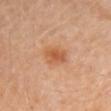This lesion was catalogued during total-body skin photography and was not selected for biopsy. On the abdomen. A male subject, about 60 years old. An algorithmic analysis of the crop reported an area of roughly 6 mm², an eccentricity of roughly 0.75, and a shape-asymmetry score of about 0.25 (0 = symmetric). The software also gave a lesion color around L≈54 a*≈24 b*≈36 in CIELAB and a lesion–skin lightness drop of about 9. A region of skin cropped from a whole-body photographic capture, roughly 15 mm wide. Longest diameter approximately 3 mm. This is a cross-polarized tile.